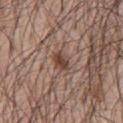The lesion was photographed on a routine skin check and not biopsied; there is no pathology result.